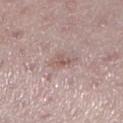{
  "biopsy_status": "not biopsied; imaged during a skin examination",
  "automated_metrics": {
    "area_mm2_approx": 3.5,
    "eccentricity": 0.8,
    "shape_asymmetry": 0.35,
    "color_variation_0_10": 2.0,
    "peripheral_color_asymmetry": 1.0,
    "nevus_likeness_0_100": 0,
    "lesion_detection_confidence_0_100": 90
  },
  "site": "leg",
  "lesion_size": {
    "long_diameter_mm_approx": 3.0
  },
  "patient": {
    "sex": "female",
    "age_approx": 45
  },
  "image": {
    "source": "total-body photography crop",
    "field_of_view_mm": 15
  },
  "lighting": "white-light"
}